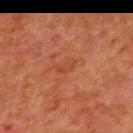Q: Was this lesion biopsied?
A: imaged on a skin check; not biopsied
Q: What is the imaging modality?
A: total-body-photography crop, ~15 mm field of view
Q: What are the patient's age and sex?
A: male, aged 78–82
Q: Lesion location?
A: the back